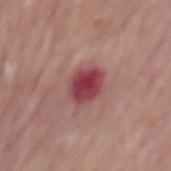Notes:
– notes: catalogued during a skin exam; not biopsied
– lesion diameter: ≈3.5 mm
– image-analysis metrics: an area of roughly 9 mm² and a shape-asymmetry score of about 0.15 (0 = symmetric)
– location: the mid back
– imaging modality: ~15 mm crop, total-body skin-cancer survey
– patient: male, aged 73 to 77
– tile lighting: white-light